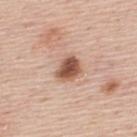Background:
On the upper back. A 15 mm crop from a total-body photograph taken for skin-cancer surveillance. A male patient, roughly 45 years of age.